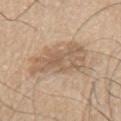<record>
  <automated_metrics>
    <area_mm2_approx>23.0</area_mm2_approx>
    <eccentricity>0.85</eccentricity>
    <shape_asymmetry>0.35</shape_asymmetry>
    <border_irregularity_0_10>4.5</border_irregularity_0_10>
    <color_variation_0_10>4.0</color_variation_0_10>
    <peripheral_color_asymmetry>1.5</peripheral_color_asymmetry>
    <lesion_detection_confidence_0_100>100</lesion_detection_confidence_0_100>
  </automated_metrics>
  <image>
    <source>total-body photography crop</source>
    <field_of_view_mm>15</field_of_view_mm>
  </image>
  <lesion_size>
    <long_diameter_mm_approx>7.5</long_diameter_mm_approx>
  </lesion_size>
  <lighting>white-light</lighting>
  <patient>
    <sex>male</sex>
    <age_approx>75</age_approx>
  </patient>
  <site>left upper arm</site>
</record>